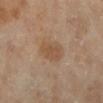Impression:
This lesion was catalogued during total-body skin photography and was not selected for biopsy.
Context:
Imaged with cross-polarized lighting. Approximately 3.5 mm at its widest. An algorithmic analysis of the crop reported a border-irregularity index near 1.5/10, a within-lesion color-variation index near 2/10, and a peripheral color-asymmetry measure near 0.5. On the left lower leg. A female patient approximately 70 years of age. A close-up tile cropped from a whole-body skin photograph, about 15 mm across.